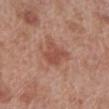{"biopsy_status": "not biopsied; imaged during a skin examination", "patient": {"sex": "male", "age_approx": 70}, "site": "left lower leg", "automated_metrics": {"area_mm2_approx": 7.0, "eccentricity": 0.65, "shape_asymmetry": 0.35, "vs_skin_contrast_norm": 6.5}, "image": {"source": "total-body photography crop", "field_of_view_mm": 15}, "lighting": "white-light", "lesion_size": {"long_diameter_mm_approx": 3.5}}